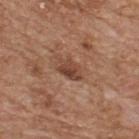Imaged during a routine full-body skin examination; the lesion was not biopsied and no histopathology is available. On the upper back. A male patient about 65 years old. A 15 mm close-up tile from a total-body photography series done for melanoma screening. Measured at roughly 3.5 mm in maximum diameter. Imaged with white-light lighting. An algorithmic analysis of the crop reported a footprint of about 5 mm², an eccentricity of roughly 0.8, and a symmetry-axis asymmetry near 0.25. The analysis additionally found an average lesion color of about L≈44 a*≈22 b*≈29 (CIELAB) and a lesion–skin lightness drop of about 10. And it measured internal color variation of about 4 on a 0–10 scale and peripheral color asymmetry of about 1.5.